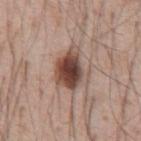Recorded during total-body skin imaging; not selected for excision or biopsy. The subject is a male aged 53–57. Captured under white-light illumination. Approximately 5 mm at its widest. On the abdomen. A region of skin cropped from a whole-body photographic capture, roughly 15 mm wide.